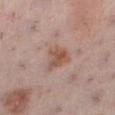workup: no biopsy performed (imaged during a skin exam); image source: ~15 mm crop, total-body skin-cancer survey; subject: female, aged approximately 55; body site: the left lower leg; size: about 3.5 mm.